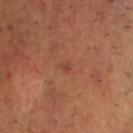The lesion was tiled from a total-body skin photograph and was not biopsied.
About 1 mm across.
Located on the front of the torso.
The tile uses cross-polarized illumination.
A male subject, aged 63 to 67.
The lesion-visualizer software estimated an area of roughly 1 mm². And it measured internal color variation of about 0 on a 0–10 scale and radial color variation of about 0. And it measured a classifier nevus-likeness of about 0/100 and a detector confidence of about 100 out of 100 that the crop contains a lesion.
A close-up tile cropped from a whole-body skin photograph, about 15 mm across.